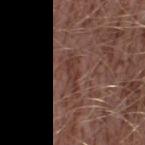Imaged during a routine full-body skin examination; the lesion was not biopsied and no histopathology is available. About 3.5 mm across. A male patient, about 45 years old. Located on the right thigh. Captured under white-light illumination. A lesion tile, about 15 mm wide, cut from a 3D total-body photograph. An algorithmic analysis of the crop reported a footprint of about 4 mm², a shape eccentricity near 0.95, and a shape-asymmetry score of about 0.45 (0 = symmetric). The software also gave internal color variation of about 0 on a 0–10 scale and a peripheral color-asymmetry measure near 0.